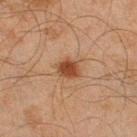No biopsy was performed on this lesion — it was imaged during a full skin examination and was not determined to be concerning. Imaged with cross-polarized lighting. A male patient, in their mid- to late 40s. From the right lower leg. The recorded lesion diameter is about 3 mm. A lesion tile, about 15 mm wide, cut from a 3D total-body photograph.